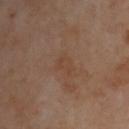| key | value |
|---|---|
| notes | total-body-photography surveillance lesion; no biopsy |
| lesion size | about 2.5 mm |
| body site | the upper back |
| image source | total-body-photography crop, ~15 mm field of view |
| image-analysis metrics | an area of roughly 3.5 mm², an eccentricity of roughly 0.75, and a shape-asymmetry score of about 0.45 (0 = symmetric); an average lesion color of about L≈42 a*≈18 b*≈29 (CIELAB), about 4 CIELAB-L* units darker than the surrounding skin, and a normalized lesion–skin contrast near 4.5; a border-irregularity rating of about 4.5/10, a within-lesion color-variation index near 1.5/10, and radial color variation of about 0.5; an automated nevus-likeness rating near 0 out of 100 and a detector confidence of about 100 out of 100 that the crop contains a lesion |
| subject | male, approximately 55 years of age |